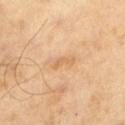Notes:
- workup — imaged on a skin check; not biopsied
- lesion size — ~2.5 mm (longest diameter)
- acquisition — ~15 mm crop, total-body skin-cancer survey
- image-analysis metrics — border irregularity of about 6 on a 0–10 scale, a within-lesion color-variation index near 0/10, and radial color variation of about 0; a classifier nevus-likeness of about 0/100 and lesion-presence confidence of about 100/100
- illumination — cross-polarized
- subject — male, aged 63–67